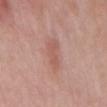Captured during whole-body skin photography for melanoma surveillance; the lesion was not biopsied. The subject is a male approximately 55 years of age. About 4 mm across. A 15 mm crop from a total-body photograph taken for skin-cancer surveillance. The lesion-visualizer software estimated about 7 CIELAB-L* units darker than the surrounding skin and a lesion-to-skin contrast of about 5.5 (normalized; higher = more distinct). The software also gave border irregularity of about 4 on a 0–10 scale and peripheral color asymmetry of about 0.5. The lesion is located on the mid back.